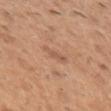Part of a total-body skin-imaging series; this lesion was reviewed on a skin check and was not flagged for biopsy. The total-body-photography lesion software estimated a nevus-likeness score of about 0/100 and lesion-presence confidence of about 100/100. A male patient, in their 40s. Imaged with white-light lighting. A 15 mm close-up tile from a total-body photography series done for melanoma screening. Located on the left upper arm. Longest diameter approximately 2.5 mm.